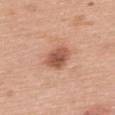biopsy_status: not biopsied; imaged during a skin examination
site: upper back
patient:
  sex: female
  age_approx: 60
image:
  source: total-body photography crop
  field_of_view_mm: 15